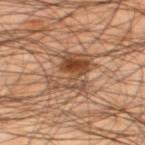Q: Was this lesion biopsied?
A: no biopsy performed (imaged during a skin exam)
Q: What is the imaging modality?
A: ~15 mm crop, total-body skin-cancer survey
Q: Who is the patient?
A: male, approximately 45 years of age
Q: Where on the body is the lesion?
A: the left thigh